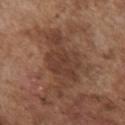Impression:
The lesion was photographed on a routine skin check and not biopsied; there is no pathology result.
Context:
The total-body-photography lesion software estimated a lesion color around L≈39 a*≈19 b*≈26 in CIELAB. The software also gave an automated nevus-likeness rating near 0 out of 100 and lesion-presence confidence of about 75/100. From the chest. A male subject, about 75 years old. This image is a 15 mm lesion crop taken from a total-body photograph.